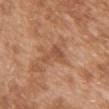Notes:
• notes — imaged on a skin check; not biopsied
• patient — female, aged approximately 30
• diameter — ~3.5 mm (longest diameter)
• image-analysis metrics — a lesion-to-skin contrast of about 5.5 (normalized; higher = more distinct); a classifier nevus-likeness of about 0/100 and lesion-presence confidence of about 100/100
• anatomic site — the chest
• tile lighting — white-light illumination
• acquisition — total-body-photography crop, ~15 mm field of view